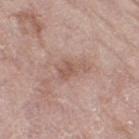Measured at roughly 3.5 mm in maximum diameter. The total-body-photography lesion software estimated an area of roughly 4.5 mm², a shape eccentricity near 0.8, and a shape-asymmetry score of about 0.4 (0 = symmetric). And it measured roughly 8 lightness units darker than nearby skin and a normalized border contrast of about 5.5. The analysis additionally found a color-variation rating of about 1.5/10. This image is a 15 mm lesion crop taken from a total-body photograph. The lesion is on the right thigh. A female subject, aged approximately 70. The tile uses white-light illumination.